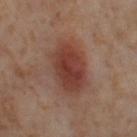image source: total-body-photography crop, ~15 mm field of view; location: the right thigh; tile lighting: cross-polarized; TBP lesion metrics: an average lesion color of about L≈41 a*≈23 b*≈26 (CIELAB), about 11 CIELAB-L* units darker than the surrounding skin, and a normalized lesion–skin contrast near 8.5; patient: female, in their mid-50s; size: about 7 mm.